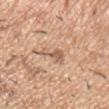No biopsy was performed on this lesion — it was imaged during a full skin examination and was not determined to be concerning.
On the left upper arm.
This is a white-light tile.
A region of skin cropped from a whole-body photographic capture, roughly 15 mm wide.
A male subject, aged 38 to 42.
Measured at roughly 3 mm in maximum diameter.